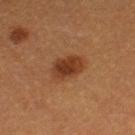notes: imaged on a skin check; not biopsied
image source: ~15 mm tile from a whole-body skin photo
lesion size: ~4 mm (longest diameter)
site: the left thigh
illumination: cross-polarized illumination
patient: female, aged 38–42
TBP lesion metrics: an eccentricity of roughly 0.8 and a symmetry-axis asymmetry near 0.15; a border-irregularity rating of about 1.5/10, a color-variation rating of about 3.5/10, and radial color variation of about 1